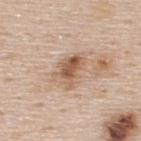Case summary:
* biopsy status · no biopsy performed (imaged during a skin exam)
* acquisition · 15 mm crop, total-body photography
* illumination · white-light
* patient · male, approximately 45 years of age
* anatomic site · the upper back
* lesion diameter · ~4.5 mm (longest diameter)